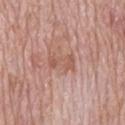Case summary:
• notes — catalogued during a skin exam; not biopsied